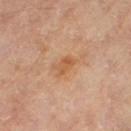The tile uses cross-polarized illumination.
The lesion's longest dimension is about 2.5 mm.
The lesion is located on the leg.
A female subject aged around 50.
A roughly 15 mm field-of-view crop from a total-body skin photograph.